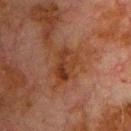| key | value |
|---|---|
| follow-up | total-body-photography surveillance lesion; no biopsy |
| patient | male, in their 80s |
| anatomic site | the chest |
| lesion size | about 4.5 mm |
| lighting | cross-polarized illumination |
| image source | total-body-photography crop, ~15 mm field of view |